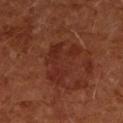Captured during whole-body skin photography for melanoma surveillance; the lesion was not biopsied. Cropped from a whole-body photographic skin survey; the tile spans about 15 mm. A female patient, in their mid-60s. The lesion is located on the left forearm. This is a cross-polarized tile.